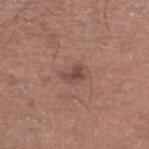The lesion was photographed on a routine skin check and not biopsied; there is no pathology result. The total-body-photography lesion software estimated border irregularity of about 4.5 on a 0–10 scale, a color-variation rating of about 2/10, and a peripheral color-asymmetry measure near 0.5. On the left lower leg. The subject is a male approximately 65 years of age. A 15 mm crop from a total-body photograph taken for skin-cancer surveillance. The tile uses white-light illumination.